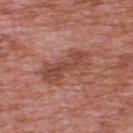Case summary:
– notes: no biopsy performed (imaged during a skin exam)
– anatomic site: the upper back
– acquisition: total-body-photography crop, ~15 mm field of view
– patient: male, aged 68 to 72